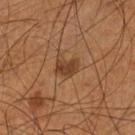No biopsy was performed on this lesion — it was imaged during a full skin examination and was not determined to be concerning.
The recorded lesion diameter is about 3 mm.
The lesion is located on the left lower leg.
A 15 mm crop from a total-body photograph taken for skin-cancer surveillance.
The patient is a male aged around 55.
The tile uses cross-polarized illumination.